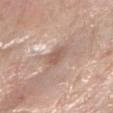Part of a total-body skin-imaging series; this lesion was reviewed on a skin check and was not flagged for biopsy. Located on the left forearm. A female patient in their mid- to late 60s. Automated image analysis of the tile measured an eccentricity of roughly 0.5 and a symmetry-axis asymmetry near 0.25. It also reported a classifier nevus-likeness of about 0/100 and a lesion-detection confidence of about 100/100. The recorded lesion diameter is about 2.5 mm. A roughly 15 mm field-of-view crop from a total-body skin photograph.